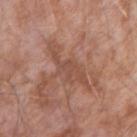Q: Is there a histopathology result?
A: no biopsy performed (imaged during a skin exam)
Q: Patient demographics?
A: male, roughly 75 years of age
Q: Lesion location?
A: the right upper arm
Q: What kind of image is this?
A: ~15 mm tile from a whole-body skin photo